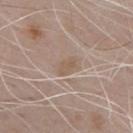Recorded during total-body skin imaging; not selected for excision or biopsy. The tile uses white-light illumination. The subject is a male approximately 70 years of age. On the chest. The recorded lesion diameter is about 2.5 mm. A 15 mm close-up tile from a total-body photography series done for melanoma screening.